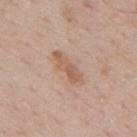<record>
  <biopsy_status>not biopsied; imaged during a skin examination</biopsy_status>
  <lighting>white-light</lighting>
  <lesion_size>
    <long_diameter_mm_approx>4.5</long_diameter_mm_approx>
  </lesion_size>
  <image>
    <source>total-body photography crop</source>
    <field_of_view_mm>15</field_of_view_mm>
  </image>
  <site>upper back</site>
  <patient>
    <sex>male</sex>
    <age_approx>70</age_approx>
  </patient>
</record>